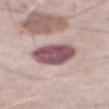notes: no biopsy performed (imaged during a skin exam); location: the abdomen; image: total-body-photography crop, ~15 mm field of view; subject: male, approximately 75 years of age.